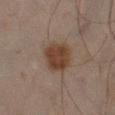Q: Was a biopsy performed?
A: catalogued during a skin exam; not biopsied
Q: What lighting was used for the tile?
A: cross-polarized illumination
Q: Lesion location?
A: the left lower leg
Q: Who is the patient?
A: male, aged 43 to 47
Q: How was this image acquired?
A: ~15 mm tile from a whole-body skin photo
Q: Lesion size?
A: ≈4 mm